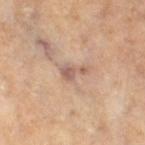The lesion was tiled from a total-body skin photograph and was not biopsied. The lesion is located on the right thigh. A 15 mm close-up tile from a total-body photography series done for melanoma screening. The patient is a female aged 48–52.